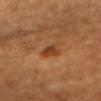workup = total-body-photography surveillance lesion; no biopsy
size = ≈2.5 mm
tile lighting = cross-polarized illumination
acquisition = ~15 mm crop, total-body skin-cancer survey
automated metrics = border irregularity of about 3 on a 0–10 scale, internal color variation of about 2.5 on a 0–10 scale, and radial color variation of about 1; a nevus-likeness score of about 75/100 and lesion-presence confidence of about 100/100
site = the head or neck
patient = female, in their mid-50s